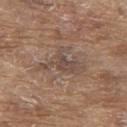Clinical impression:
This lesion was catalogued during total-body skin photography and was not selected for biopsy.
Image and clinical context:
This is a white-light tile. This image is a 15 mm lesion crop taken from a total-body photograph. The patient is a male aged approximately 80. About 5.5 mm across. Automated tile analysis of the lesion measured a lesion area of about 11 mm², an outline eccentricity of about 0.8 (0 = round, 1 = elongated), and two-axis asymmetry of about 0.6. The software also gave border irregularity of about 9 on a 0–10 scale, a color-variation rating of about 2.5/10, and radial color variation of about 0.5. From the back.